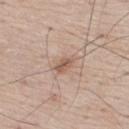<record>
<biopsy_status>not biopsied; imaged during a skin examination</biopsy_status>
<automated_metrics>
  <area_mm2_approx>3.5</area_mm2_approx>
  <eccentricity>0.8</eccentricity>
  <shape_asymmetry>0.2</shape_asymmetry>
  <cielab_L>57</cielab_L>
  <cielab_a>18</cielab_a>
  <cielab_b>28</cielab_b>
  <vs_skin_darker_L>10.0</vs_skin_darker_L>
  <vs_skin_contrast_norm>7.0</vs_skin_contrast_norm>
  <nevus_likeness_0_100>15</nevus_likeness_0_100>
  <lesion_detection_confidence_0_100>100</lesion_detection_confidence_0_100>
</automated_metrics>
<site>upper back</site>
<lesion_size>
  <long_diameter_mm_approx>2.5</long_diameter_mm_approx>
</lesion_size>
<lighting>white-light</lighting>
<image>
  <source>total-body photography crop</source>
  <field_of_view_mm>15</field_of_view_mm>
</image>
<patient>
  <sex>male</sex>
  <age_approx>75</age_approx>
</patient>
</record>